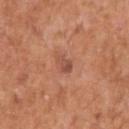The lesion was photographed on a routine skin check and not biopsied; there is no pathology result.
A close-up tile cropped from a whole-body skin photograph, about 15 mm across.
Captured under white-light illumination.
The lesion is located on the right upper arm.
A male subject, aged 73 to 77.
The recorded lesion diameter is about 2.5 mm.